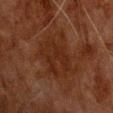<tbp_lesion>
<lesion_size>
  <long_diameter_mm_approx>5.5</long_diameter_mm_approx>
</lesion_size>
<image>
  <source>total-body photography crop</source>
  <field_of_view_mm>15</field_of_view_mm>
</image>
<automated_metrics>
  <area_mm2_approx>20.0</area_mm2_approx>
  <eccentricity>0.55</eccentricity>
  <shape_asymmetry>0.3</shape_asymmetry>
  <cielab_L>21</cielab_L>
  <cielab_a>18</cielab_a>
  <cielab_b>24</cielab_b>
  <vs_skin_darker_L>4.0</vs_skin_darker_L>
  <vs_skin_contrast_norm>6.0</vs_skin_contrast_norm>
  <lesion_detection_confidence_0_100>95</lesion_detection_confidence_0_100>
</automated_metrics>
<site>back</site>
<patient>
  <sex>male</sex>
  <age_approx>80</age_approx>
</patient>
</tbp_lesion>